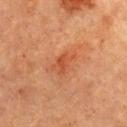No biopsy was performed on this lesion — it was imaged during a full skin examination and was not determined to be concerning. The lesion is located on the chest. Approximately 3.5 mm at its widest. An algorithmic analysis of the crop reported a lesion area of about 6 mm², an outline eccentricity of about 0.8 (0 = round, 1 = elongated), and a shape-asymmetry score of about 0.35 (0 = symmetric). The software also gave border irregularity of about 3.5 on a 0–10 scale, a color-variation rating of about 3/10, and a peripheral color-asymmetry measure near 1. A female patient aged 58 to 62. A roughly 15 mm field-of-view crop from a total-body skin photograph. Captured under cross-polarized illumination.